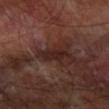Recorded during total-body skin imaging; not selected for excision or biopsy. This image is a 15 mm lesion crop taken from a total-body photograph. A male patient approximately 65 years of age. The tile uses cross-polarized illumination. Automated tile analysis of the lesion measured about 7 CIELAB-L* units darker than the surrounding skin and a lesion-to-skin contrast of about 8.5 (normalized; higher = more distinct). And it measured border irregularity of about 3 on a 0–10 scale, a within-lesion color-variation index near 2/10, and peripheral color asymmetry of about 1. It also reported a nevus-likeness score of about 0/100 and lesion-presence confidence of about 65/100. From the right forearm.